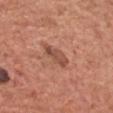Impression: Captured during whole-body skin photography for melanoma surveillance; the lesion was not biopsied. Acquisition and patient details: A 15 mm close-up extracted from a 3D total-body photography capture. On the head or neck. The subject is a male aged 68–72.